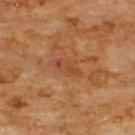Notes:
* workup — total-body-photography surveillance lesion; no biopsy
* image source — ~15 mm tile from a whole-body skin photo
* patient — male, aged 58 to 62
* anatomic site — the front of the torso
* TBP lesion metrics — a footprint of about 3 mm² and an outline eccentricity of about 0.95 (0 = round, 1 = elongated); a lesion color around L≈44 a*≈26 b*≈35 in CIELAB, about 7 CIELAB-L* units darker than the surrounding skin, and a normalized lesion–skin contrast near 5.5; border irregularity of about 3.5 on a 0–10 scale and internal color variation of about 0 on a 0–10 scale; a classifier nevus-likeness of about 0/100
* lesion diameter — ~3 mm (longest diameter)